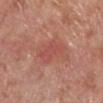notes = total-body-photography surveillance lesion; no biopsy
patient = male, approximately 80 years of age
body site = the right lower leg
image source = ~15 mm tile from a whole-body skin photo